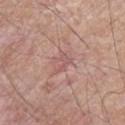Q: Was this lesion biopsied?
A: catalogued during a skin exam; not biopsied
Q: What is the imaging modality?
A: total-body-photography crop, ~15 mm field of view
Q: Patient demographics?
A: male, aged approximately 60
Q: Lesion location?
A: the upper back
Q: How was the tile lit?
A: white-light illumination